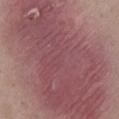Captured during whole-body skin photography for melanoma surveillance; the lesion was not biopsied. Automated image analysis of the tile measured border irregularity of about 4 on a 0–10 scale and radial color variation of about 1.5. This image is a 15 mm lesion crop taken from a total-body photograph. Imaged with white-light lighting. A female patient, approximately 35 years of age. The lesion is located on the chest.